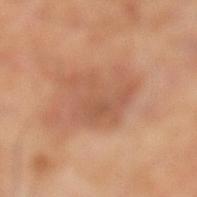Clinical impression:
The lesion was photographed on a routine skin check and not biopsied; there is no pathology result.